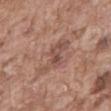{
  "biopsy_status": "not biopsied; imaged during a skin examination",
  "patient": {
    "sex": "male",
    "age_approx": 75
  },
  "site": "abdomen",
  "lighting": "white-light",
  "automated_metrics": {
    "cielab_L": 50,
    "cielab_a": 20,
    "cielab_b": 25,
    "vs_skin_darker_L": 8.0,
    "vs_skin_contrast_norm": 6.0,
    "border_irregularity_0_10": 7.0,
    "color_variation_0_10": 4.0,
    "peripheral_color_asymmetry": 1.5
  },
  "lesion_size": {
    "long_diameter_mm_approx": 5.5
  },
  "image": {
    "source": "total-body photography crop",
    "field_of_view_mm": 15
  }
}